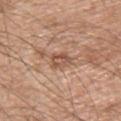Part of a total-body skin-imaging series; this lesion was reviewed on a skin check and was not flagged for biopsy. A male patient aged 53–57. On the back. A 15 mm close-up tile from a total-body photography series done for melanoma screening.